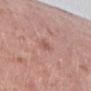biopsy_status: not biopsied; imaged during a skin examination
patient:
  sex: female
  age_approx: 50
site: leg
lighting: white-light
image:
  source: total-body photography crop
  field_of_view_mm: 15